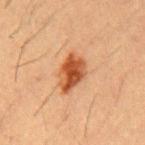follow-up: imaged on a skin check; not biopsied
image: ~15 mm tile from a whole-body skin photo
body site: the chest
tile lighting: cross-polarized
size: about 4.5 mm
automated metrics: a lesion area of about 9.5 mm², a shape eccentricity near 0.75, and a shape-asymmetry score of about 0.25 (0 = symmetric); a mean CIELAB color near L≈46 a*≈26 b*≈36, roughly 14 lightness units darker than nearby skin, and a lesion-to-skin contrast of about 10.5 (normalized; higher = more distinct); an automated nevus-likeness rating near 100 out of 100 and lesion-presence confidence of about 100/100
patient: male, about 55 years old